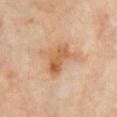biopsy status = imaged on a skin check; not biopsied
automated metrics = an area of roughly 9.5 mm²; an average lesion color of about L≈60 a*≈22 b*≈38 (CIELAB) and a normalized border contrast of about 8
location = the chest
patient = female, about 60 years old
image source = ~15 mm crop, total-body skin-cancer survey